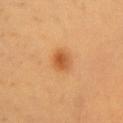patient: female, aged 58 to 62 | site: the front of the torso | lesion size: ~3 mm (longest diameter) | image-analysis metrics: a border-irregularity index near 2/10, a within-lesion color-variation index near 4/10, and a peripheral color-asymmetry measure near 1.5; a classifier nevus-likeness of about 95/100 and a detector confidence of about 100 out of 100 that the crop contains a lesion | imaging modality: 15 mm crop, total-body photography.